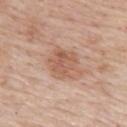Part of a total-body skin-imaging series; this lesion was reviewed on a skin check and was not flagged for biopsy.
A female patient, aged approximately 70.
Approximately 4 mm at its widest.
A 15 mm close-up extracted from a 3D total-body photography capture.
From the upper back.